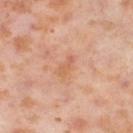  biopsy_status: not biopsied; imaged during a skin examination
  site: right thigh
  patient:
    sex: female
    age_approx: 55
  lighting: cross-polarized
  lesion_size:
    long_diameter_mm_approx: 3.0
  image:
    source: total-body photography crop
    field_of_view_mm: 15